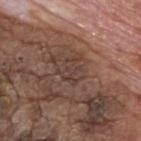| field | value |
|---|---|
| workup | no biopsy performed (imaged during a skin exam) |
| anatomic site | the upper back |
| image | 15 mm crop, total-body photography |
| image-analysis metrics | a lesion area of about 5.5 mm², an eccentricity of roughly 0.6, and a shape-asymmetry score of about 0.4 (0 = symmetric); a mean CIELAB color near L≈39 a*≈17 b*≈22 and a normalized border contrast of about 5; a classifier nevus-likeness of about 0/100 and lesion-presence confidence of about 65/100 |
| patient | male, about 75 years old |
| tile lighting | white-light |